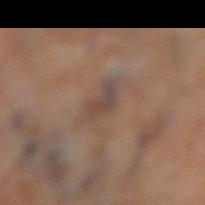{
  "biopsy_status": "not biopsied; imaged during a skin examination",
  "lighting": "cross-polarized",
  "automated_metrics": {
    "border_irregularity_0_10": 4.0,
    "color_variation_0_10": 3.5,
    "peripheral_color_asymmetry": 1.0
  },
  "image": {
    "source": "total-body photography crop",
    "field_of_view_mm": 15
  },
  "lesion_size": {
    "long_diameter_mm_approx": 3.5
  },
  "site": "right lower leg"
}